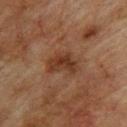Q: Illumination type?
A: cross-polarized illumination
Q: How was this image acquired?
A: total-body-photography crop, ~15 mm field of view
Q: Lesion location?
A: the upper back
Q: Who is the patient?
A: male, roughly 75 years of age
Q: Lesion size?
A: ≈4 mm
Q: What did automated image analysis measure?
A: a lesion area of about 8 mm², an outline eccentricity of about 0.75 (0 = round, 1 = elongated), and two-axis asymmetry of about 0.4; a border-irregularity rating of about 4/10, a within-lesion color-variation index near 3/10, and a peripheral color-asymmetry measure near 1; an automated nevus-likeness rating near 0 out of 100 and a lesion-detection confidence of about 100/100